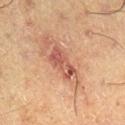Q: Was a biopsy performed?
A: no biopsy performed (imaged during a skin exam)
Q: Who is the patient?
A: male, approximately 60 years of age
Q: How was this image acquired?
A: ~15 mm tile from a whole-body skin photo
Q: What is the lesion's diameter?
A: ~4 mm (longest diameter)
Q: What did automated image analysis measure?
A: an area of roughly 7 mm², an outline eccentricity of about 0.9 (0 = round, 1 = elongated), and two-axis asymmetry of about 0.3; a lesion color around L≈42 a*≈21 b*≈24 in CIELAB, about 9 CIELAB-L* units darker than the surrounding skin, and a normalized border contrast of about 7.5; a within-lesion color-variation index near 4.5/10 and a peripheral color-asymmetry measure near 1.5; lesion-presence confidence of about 100/100
Q: What lighting was used for the tile?
A: cross-polarized
Q: Lesion location?
A: the right lower leg